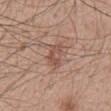  biopsy_status: not biopsied; imaged during a skin examination
  image:
    source: total-body photography crop
    field_of_view_mm: 15
  lesion_size:
    long_diameter_mm_approx: 3.0
  site: mid back
  lighting: white-light
  automated_metrics:
    area_mm2_approx: 3.5
    eccentricity: 0.85
  patient:
    sex: male
    age_approx: 50